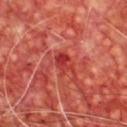Clinical impression: Part of a total-body skin-imaging series; this lesion was reviewed on a skin check and was not flagged for biopsy. Clinical summary: The patient is a male aged around 65. Located on the chest. Cropped from a whole-body photographic skin survey; the tile spans about 15 mm.